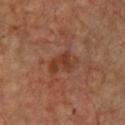Captured during whole-body skin photography for melanoma surveillance; the lesion was not biopsied. The total-body-photography lesion software estimated an area of roughly 6 mm² and an outline eccentricity of about 0.8 (0 = round, 1 = elongated). The software also gave a lesion color around L≈38 a*≈23 b*≈31 in CIELAB, about 7 CIELAB-L* units darker than the surrounding skin, and a normalized lesion–skin contrast near 7. From the chest. A region of skin cropped from a whole-body photographic capture, roughly 15 mm wide. The lesion's longest dimension is about 3.5 mm. A male subject, about 50 years old.